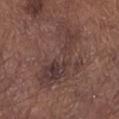Q: Was this lesion biopsied?
A: imaged on a skin check; not biopsied
Q: What kind of image is this?
A: ~15 mm tile from a whole-body skin photo
Q: How large is the lesion?
A: ~8.5 mm (longest diameter)
Q: What did automated image analysis measure?
A: an eccentricity of roughly 0.9; a lesion color around L≈37 a*≈17 b*≈19 in CIELAB, about 7 CIELAB-L* units darker than the surrounding skin, and a lesion-to-skin contrast of about 7 (normalized; higher = more distinct)
Q: What are the patient's age and sex?
A: male, in their mid- to late 50s
Q: How was the tile lit?
A: white-light
Q: Where on the body is the lesion?
A: the left lower leg